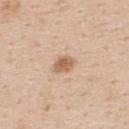The lesion was photographed on a routine skin check and not biopsied; there is no pathology result. The recorded lesion diameter is about 2.5 mm. Cropped from a total-body skin-imaging series; the visible field is about 15 mm. The lesion-visualizer software estimated an area of roughly 4.5 mm², a shape eccentricity near 0.65, and a shape-asymmetry score of about 0.2 (0 = symmetric). A female subject, aged 43 to 47. From the upper back.